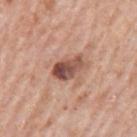The lesion was tiled from a total-body skin photograph and was not biopsied. Automated tile analysis of the lesion measured an average lesion color of about L≈50 a*≈23 b*≈26 (CIELAB), about 15 CIELAB-L* units darker than the surrounding skin, and a lesion-to-skin contrast of about 10.5 (normalized; higher = more distinct). And it measured a border-irregularity index near 2.5/10, internal color variation of about 9 on a 0–10 scale, and peripheral color asymmetry of about 3.5. A male patient approximately 80 years of age. Cropped from a whole-body photographic skin survey; the tile spans about 15 mm. Approximately 3.5 mm at its widest. The lesion is located on the left upper arm.